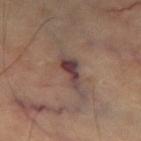biopsy status=total-body-photography surveillance lesion; no biopsy | automated metrics=a lesion area of about 6 mm², a shape eccentricity near 0.9, and two-axis asymmetry of about 0.35 | lighting=cross-polarized illumination | acquisition=15 mm crop, total-body photography | location=the left thigh | subject=male, about 65 years old.